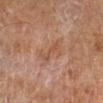Assessment:
No biopsy was performed on this lesion — it was imaged during a full skin examination and was not determined to be concerning.